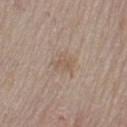{
  "biopsy_status": "not biopsied; imaged during a skin examination",
  "automated_metrics": {
    "cielab_L": 56,
    "cielab_a": 14,
    "cielab_b": 26,
    "vs_skin_darker_L": 6.0,
    "vs_skin_contrast_norm": 5.0
  },
  "lighting": "white-light",
  "image": {
    "source": "total-body photography crop",
    "field_of_view_mm": 15
  },
  "lesion_size": {
    "long_diameter_mm_approx": 2.5
  },
  "patient": {
    "sex": "male",
    "age_approx": 75
  },
  "site": "arm"
}